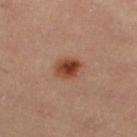<lesion>
<biopsy_status>not biopsied; imaged during a skin examination</biopsy_status>
<patient>
  <sex>female</sex>
  <age_approx>35</age_approx>
</patient>
<lesion_size>
  <long_diameter_mm_approx>3.0</long_diameter_mm_approx>
</lesion_size>
<image>
  <source>total-body photography crop</source>
  <field_of_view_mm>15</field_of_view_mm>
</image>
<site>left lower leg</site>
</lesion>